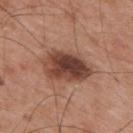Impression:
No biopsy was performed on this lesion — it was imaged during a full skin examination and was not determined to be concerning.
Image and clinical context:
Approximately 6 mm at its widest. From the mid back. This is a white-light tile. Cropped from a total-body skin-imaging series; the visible field is about 15 mm. The subject is a male roughly 55 years of age.